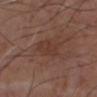{
  "biopsy_status": "not biopsied; imaged during a skin examination",
  "image": {
    "source": "total-body photography crop",
    "field_of_view_mm": 15
  },
  "lighting": "white-light",
  "lesion_size": {
    "long_diameter_mm_approx": 3.5
  },
  "automated_metrics": {
    "eccentricity": 0.75,
    "shape_asymmetry": 0.3,
    "cielab_L": 36,
    "cielab_a": 19,
    "cielab_b": 25,
    "vs_skin_contrast_norm": 5.0,
    "border_irregularity_0_10": 3.0,
    "color_variation_0_10": 1.5
  },
  "site": "chest",
  "patient": {
    "sex": "male",
    "age_approx": 75
  }
}